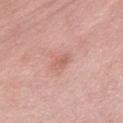Impression: Part of a total-body skin-imaging series; this lesion was reviewed on a skin check and was not flagged for biopsy. Background: A region of skin cropped from a whole-body photographic capture, roughly 15 mm wide. The lesion is located on the abdomen. Imaged with white-light lighting. The subject is a male aged approximately 55. Measured at roughly 3 mm in maximum diameter.